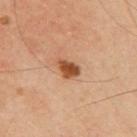size: ≈3 mm
image: 15 mm crop, total-body photography
site: the upper back
tile lighting: cross-polarized illumination
subject: male, aged around 35